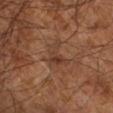Findings:
- biopsy status: no biopsy performed (imaged during a skin exam)
- subject: approximately 65 years of age
- lighting: cross-polarized illumination
- image source: ~15 mm tile from a whole-body skin photo
- location: the leg
- automated metrics: a mean CIELAB color near L≈38 a*≈19 b*≈28, roughly 6 lightness units darker than nearby skin, and a normalized border contrast of about 5.5
- lesion diameter: about 3.5 mm